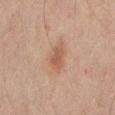{"biopsy_status": "not biopsied; imaged during a skin examination", "image": {"source": "total-body photography crop", "field_of_view_mm": 15}, "patient": {"sex": "male", "age_approx": 45}, "lighting": "cross-polarized", "automated_metrics": {"area_mm2_approx": 6.5, "eccentricity": 0.8, "shape_asymmetry": 0.25, "border_irregularity_0_10": 2.5}, "lesion_size": {"long_diameter_mm_approx": 4.0}, "site": "mid back"}